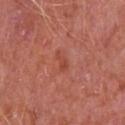biopsy status — catalogued during a skin exam; not biopsied | subject — male, in their mid- to late 60s | TBP lesion metrics — a shape-asymmetry score of about 0.55 (0 = symmetric); border irregularity of about 5 on a 0–10 scale, internal color variation of about 0 on a 0–10 scale, and a peripheral color-asymmetry measure near 0 | acquisition — total-body-photography crop, ~15 mm field of view | diameter — about 2.5 mm | location — the chest.